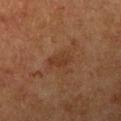Imaged during a routine full-body skin examination; the lesion was not biopsied and no histopathology is available.